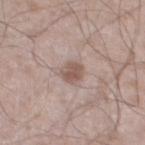Impression:
The lesion was tiled from a total-body skin photograph and was not biopsied.